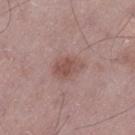Acquisition and patient details: A 15 mm crop from a total-body photograph taken for skin-cancer surveillance. The subject is a male in their 50s. The lesion is on the left thigh.